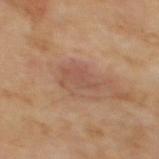Notes:
* biopsy status — no biopsy performed (imaged during a skin exam)
* diameter — about 5 mm
* imaging modality — ~15 mm crop, total-body skin-cancer survey
* anatomic site — the upper back
* illumination — cross-polarized illumination